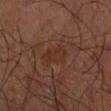Captured during whole-body skin photography for melanoma surveillance; the lesion was not biopsied.
A region of skin cropped from a whole-body photographic capture, roughly 15 mm wide.
This is a cross-polarized tile.
The lesion is located on the right upper arm.
A male patient, approximately 70 years of age.
The total-body-photography lesion software estimated an area of roughly 7 mm², a shape eccentricity near 0.85, and a shape-asymmetry score of about 0.25 (0 = symmetric). The software also gave a border-irregularity index near 3/10. It also reported a classifier nevus-likeness of about 0/100 and a detector confidence of about 90 out of 100 that the crop contains a lesion.